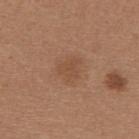Q: Is there a histopathology result?
A: catalogued during a skin exam; not biopsied